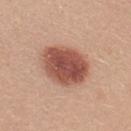Impression:
This lesion was catalogued during total-body skin photography and was not selected for biopsy.
Background:
A 15 mm crop from a total-body photograph taken for skin-cancer surveillance. The tile uses white-light illumination. The lesion is located on the upper back. Measured at roughly 6 mm in maximum diameter. A female subject, in their mid- to late 20s.